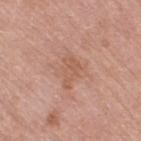Assessment:
Imaged during a routine full-body skin examination; the lesion was not biopsied and no histopathology is available.
Clinical summary:
A female subject, aged around 70. Captured under white-light illumination. The lesion's longest dimension is about 3.5 mm. An algorithmic analysis of the crop reported a footprint of about 4.5 mm², an eccentricity of roughly 0.85, and two-axis asymmetry of about 0.5. It also reported a border-irregularity index near 5.5/10, internal color variation of about 2 on a 0–10 scale, and peripheral color asymmetry of about 0.5. The lesion is on the right thigh. This image is a 15 mm lesion crop taken from a total-body photograph.